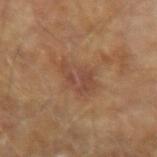Q: Was a biopsy performed?
A: total-body-photography surveillance lesion; no biopsy
Q: Where on the body is the lesion?
A: the left upper arm
Q: Patient demographics?
A: male, aged around 65
Q: What kind of image is this?
A: ~15 mm tile from a whole-body skin photo
Q: How large is the lesion?
A: ~4.5 mm (longest diameter)